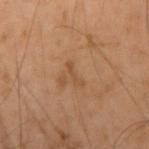Q: What are the patient's age and sex?
A: male, roughly 55 years of age
Q: How was this image acquired?
A: ~15 mm crop, total-body skin-cancer survey
Q: Lesion location?
A: the right upper arm
Q: How large is the lesion?
A: ≈3 mm
Q: Illumination type?
A: cross-polarized